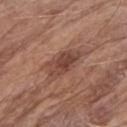| key | value |
|---|---|
| follow-up | imaged on a skin check; not biopsied |
| anatomic site | the right upper arm |
| image | 15 mm crop, total-body photography |
| diameter | ≈5.5 mm |
| subject | female, in their mid-80s or older |
| illumination | white-light illumination |